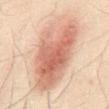Imaged during a routine full-body skin examination; the lesion was not biopsied and no histopathology is available.
The lesion's longest dimension is about 10 mm.
Automated tile analysis of the lesion measured a border-irregularity rating of about 3/10, a color-variation rating of about 6/10, and radial color variation of about 2.
On the abdomen.
A close-up tile cropped from a whole-body skin photograph, about 15 mm across.
Captured under cross-polarized illumination.
A male patient, approximately 50 years of age.